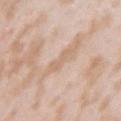Clinical impression:
Imaged during a routine full-body skin examination; the lesion was not biopsied and no histopathology is available.
Image and clinical context:
A 15 mm close-up tile from a total-body photography series done for melanoma screening. Imaged with white-light lighting. The lesion is located on the left upper arm. A female subject, roughly 25 years of age. The lesion-visualizer software estimated an outline eccentricity of about 0.9 (0 = round, 1 = elongated). Measured at roughly 3 mm in maximum diameter.